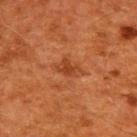workup: total-body-photography surveillance lesion; no biopsy | anatomic site: the upper back | acquisition: ~15 mm crop, total-body skin-cancer survey | subject: male, approximately 60 years of age.